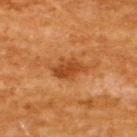Case summary:
* follow-up — imaged on a skin check; not biopsied
* patient — male, aged 58–62
* acquisition — total-body-photography crop, ~15 mm field of view
* lesion diameter — ~4.5 mm (longest diameter)
* location — the upper back